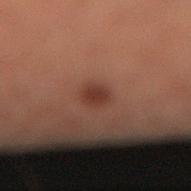A male subject, aged approximately 60. About 2.5 mm across. A region of skin cropped from a whole-body photographic capture, roughly 15 mm wide. The lesion is on the right lower leg. The tile uses cross-polarized illumination.